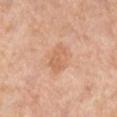Q: Was a biopsy performed?
A: catalogued during a skin exam; not biopsied
Q: What kind of image is this?
A: ~15 mm tile from a whole-body skin photo
Q: What are the patient's age and sex?
A: female, approximately 55 years of age
Q: What did automated image analysis measure?
A: an area of roughly 7 mm² and a shape eccentricity near 0.7; an automated nevus-likeness rating near 0 out of 100
Q: How large is the lesion?
A: about 3.5 mm
Q: Where on the body is the lesion?
A: the leg
Q: Illumination type?
A: cross-polarized